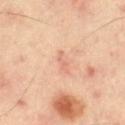Assessment:
Recorded during total-body skin imaging; not selected for excision or biopsy.
Background:
From the leg. A 15 mm close-up extracted from a 3D total-body photography capture.